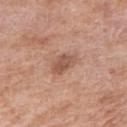<case>
  <biopsy_status>not biopsied; imaged during a skin examination</biopsy_status>
  <patient>
    <sex>female</sex>
    <age_approx>70</age_approx>
  </patient>
  <site>right upper arm</site>
  <image>
    <source>total-body photography crop</source>
    <field_of_view_mm>15</field_of_view_mm>
  </image>
</case>